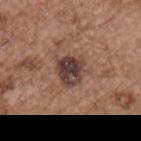follow-up = total-body-photography surveillance lesion; no biopsy
imaging modality = ~15 mm crop, total-body skin-cancer survey
lesion diameter = about 3.5 mm
lighting = white-light
TBP lesion metrics = a nevus-likeness score of about 25/100 and lesion-presence confidence of about 100/100
patient = male, approximately 55 years of age
body site = the chest